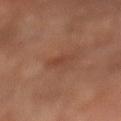The tile uses cross-polarized illumination. An algorithmic analysis of the crop reported an area of roughly 3 mm² and an eccentricity of roughly 0.8. And it measured a within-lesion color-variation index near 0.5/10 and radial color variation of about 0. And it measured a nevus-likeness score of about 0/100 and a lesion-detection confidence of about 100/100. A male patient, about 45 years old. The lesion's longest dimension is about 2.5 mm. The lesion is located on the right forearm. A 15 mm crop from a total-body photograph taken for skin-cancer surveillance.